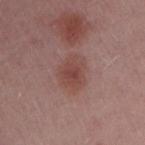The lesion was photographed on a routine skin check and not biopsied; there is no pathology result.
The subject is a female approximately 45 years of age.
Located on the arm.
A 15 mm close-up extracted from a 3D total-body photography capture.